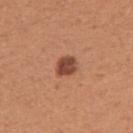patient = female, aged approximately 40 | imaging modality = total-body-photography crop, ~15 mm field of view | illumination = white-light illumination | site = the arm.